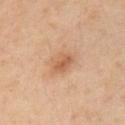Assessment:
The lesion was tiled from a total-body skin photograph and was not biopsied.
Clinical summary:
A male patient aged approximately 50. A region of skin cropped from a whole-body photographic capture, roughly 15 mm wide. The total-body-photography lesion software estimated an area of roughly 6 mm², an eccentricity of roughly 0.6, and a symmetry-axis asymmetry near 0.2. The analysis additionally found a lesion color around L≈44 a*≈17 b*≈28 in CIELAB, about 7 CIELAB-L* units darker than the surrounding skin, and a normalized border contrast of about 6. The lesion is on the front of the torso. This is a cross-polarized tile. The lesion's longest dimension is about 3 mm.